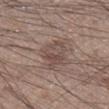Assessment:
No biopsy was performed on this lesion — it was imaged during a full skin examination and was not determined to be concerning.
Image and clinical context:
This is a white-light tile. Automated tile analysis of the lesion measured a lesion area of about 10 mm² and a shape eccentricity near 0.7. The analysis additionally found border irregularity of about 3.5 on a 0–10 scale, a within-lesion color-variation index near 4.5/10, and a peripheral color-asymmetry measure near 1.5. A 15 mm crop from a total-body photograph taken for skin-cancer surveillance. A male patient, approximately 20 years of age. On the right lower leg. Longest diameter approximately 4 mm.